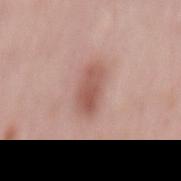| feature | finding |
|---|---|
| follow-up | no biopsy performed (imaged during a skin exam) |
| patient | female, aged approximately 65 |
| anatomic site | the mid back |
| image | ~15 mm tile from a whole-body skin photo |